This is a white-light tile. Longest diameter approximately 4.5 mm. The lesion-visualizer software estimated a classifier nevus-likeness of about 55/100 and a detector confidence of about 100 out of 100 that the crop contains a lesion. Cropped from a whole-body photographic skin survey; the tile spans about 15 mm. A male subject, about 55 years old:
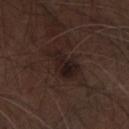{
  "diagnosis": {
    "histopathology": "seborrheic keratosis",
    "malignancy": "benign",
    "taxonomic_path": [
      "Benign",
      "Benign epidermal proliferations",
      "Seborrheic keratosis"
    ]
  }
}